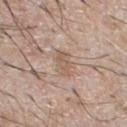Imaged during a routine full-body skin examination; the lesion was not biopsied and no histopathology is available. The lesion is located on the front of the torso. A male subject in their mid- to late 60s. A 15 mm crop from a total-body photograph taken for skin-cancer surveillance.